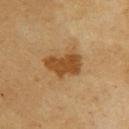The lesion was photographed on a routine skin check and not biopsied; there is no pathology result. A 15 mm crop from a total-body photograph taken for skin-cancer surveillance. Measured at roughly 4.5 mm in maximum diameter. The lesion is on the upper back. The subject is a female aged 58–62. Captured under cross-polarized illumination. An algorithmic analysis of the crop reported a lesion area of about 9.5 mm², an eccentricity of roughly 0.8, and two-axis asymmetry of about 0.35. And it measured a classifier nevus-likeness of about 95/100 and lesion-presence confidence of about 100/100.